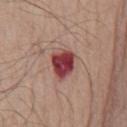Recorded during total-body skin imaging; not selected for excision or biopsy.
The patient is a male in their mid- to late 70s.
A roughly 15 mm field-of-view crop from a total-body skin photograph.
Captured under white-light illumination.
The lesion is located on the chest.